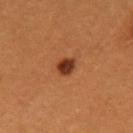workup: no biopsy performed (imaged during a skin exam) | image source: total-body-photography crop, ~15 mm field of view | site: the left upper arm | lesion size: ~2.5 mm (longest diameter) | tile lighting: cross-polarized illumination | automated metrics: a lesion color around L≈34 a*≈25 b*≈33 in CIELAB, a lesion–skin lightness drop of about 14, and a lesion-to-skin contrast of about 12 (normalized; higher = more distinct); a within-lesion color-variation index near 3/10 and radial color variation of about 1 | patient: female, about 30 years old.